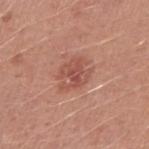{
  "biopsy_status": "not biopsied; imaged during a skin examination",
  "lighting": "white-light",
  "patient": {
    "sex": "male",
    "age_approx": 25
  },
  "lesion_size": {
    "long_diameter_mm_approx": 4.0
  },
  "automated_metrics": {
    "area_mm2_approx": 9.0,
    "eccentricity": 0.6,
    "cielab_L": 52,
    "cielab_a": 26,
    "cielab_b": 28,
    "vs_skin_darker_L": 9.0,
    "vs_skin_contrast_norm": 6.0,
    "nevus_likeness_0_100": 5
  },
  "image": {
    "source": "total-body photography crop",
    "field_of_view_mm": 15
  },
  "site": "right upper arm"
}